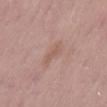The lesion was photographed on a routine skin check and not biopsied; there is no pathology result.